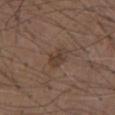notes = catalogued during a skin exam; not biopsied
tile lighting = white-light
location = the chest
image source = ~15 mm crop, total-body skin-cancer survey
lesion diameter = ~3 mm (longest diameter)
patient = male, in their mid- to late 70s